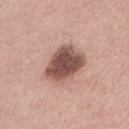Part of a total-body skin-imaging series; this lesion was reviewed on a skin check and was not flagged for biopsy. The lesion-visualizer software estimated an area of roughly 15 mm² and a shape eccentricity near 0.6. And it measured a classifier nevus-likeness of about 20/100. On the back. A region of skin cropped from a whole-body photographic capture, roughly 15 mm wide. The subject is a male aged 53 to 57.